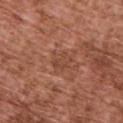Assessment: The lesion was photographed on a routine skin check and not biopsied; there is no pathology result. Context: Located on the upper back. The tile uses white-light illumination. The patient is a male aged 73–77. The lesion-visualizer software estimated an average lesion color of about L≈46 a*≈24 b*≈31 (CIELAB), a lesion–skin lightness drop of about 6, and a normalized border contrast of about 5. And it measured a nevus-likeness score of about 0/100 and a detector confidence of about 100 out of 100 that the crop contains a lesion. Measured at roughly 3 mm in maximum diameter. This image is a 15 mm lesion crop taken from a total-body photograph.